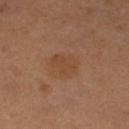size: ≈3 mm; subject: female, in their 30s; body site: the left thigh; illumination: cross-polarized; image source: ~15 mm crop, total-body skin-cancer survey.